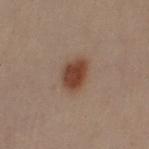This lesion was catalogued during total-body skin photography and was not selected for biopsy. Located on the left upper arm. A male subject, aged 48–52. This is a cross-polarized tile. About 4 mm across. A 15 mm close-up tile from a total-body photography series done for melanoma screening.